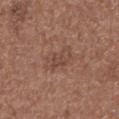workup = catalogued during a skin exam; not biopsied | body site = the left lower leg | acquisition = total-body-photography crop, ~15 mm field of view | subject = male, aged 73–77.